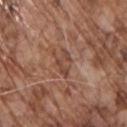Q: How large is the lesion?
A: ~3 mm (longest diameter)
Q: What is the anatomic site?
A: the chest
Q: What kind of image is this?
A: ~15 mm crop, total-body skin-cancer survey
Q: Who is the patient?
A: male, roughly 70 years of age
Q: What lighting was used for the tile?
A: white-light illumination